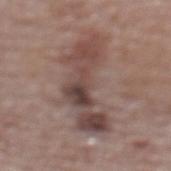- subject: female, in their mid- to late 60s
- anatomic site: the upper back
- image: ~15 mm tile from a whole-body skin photo
- diameter: about 9 mm
- automated lesion analysis: a footprint of about 23 mm², a shape eccentricity near 0.95, and a shape-asymmetry score of about 0.55 (0 = symmetric); a mean CIELAB color near L≈44 a*≈17 b*≈20, about 11 CIELAB-L* units darker than the surrounding skin, and a lesion-to-skin contrast of about 8.5 (normalized; higher = more distinct); a within-lesion color-variation index near 8/10; a nevus-likeness score of about 0/100 and a lesion-detection confidence of about 90/100